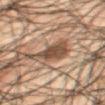This lesion was catalogued during total-body skin photography and was not selected for biopsy. The tile uses cross-polarized illumination. Measured at roughly 4.5 mm in maximum diameter. The subject is a male aged 48–52. The lesion is located on the mid back. A close-up tile cropped from a whole-body skin photograph, about 15 mm across.